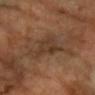{
  "biopsy_status": "not biopsied; imaged during a skin examination",
  "image": {
    "source": "total-body photography crop",
    "field_of_view_mm": 15
  },
  "automated_metrics": {
    "cielab_L": 37,
    "cielab_a": 17,
    "cielab_b": 29,
    "vs_skin_darker_L": 6.0,
    "vs_skin_contrast_norm": 5.5
  },
  "patient": {
    "sex": "female",
    "age_approx": 60
  },
  "lighting": "cross-polarized",
  "site": "left arm"
}